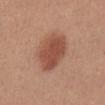Impression: Part of a total-body skin-imaging series; this lesion was reviewed on a skin check and was not flagged for biopsy. Acquisition and patient details: The tile uses white-light illumination. Approximately 5.5 mm at its widest. A female patient, in their mid- to late 40s. A region of skin cropped from a whole-body photographic capture, roughly 15 mm wide. The lesion is located on the abdomen.